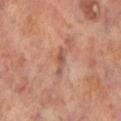No biopsy was performed on this lesion — it was imaged during a full skin examination and was not determined to be concerning.
The patient is a male approximately 70 years of age.
A roughly 15 mm field-of-view crop from a total-body skin photograph.
From the left lower leg.